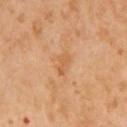Findings:
– biopsy status — total-body-photography surveillance lesion; no biopsy
– automated metrics — a normalized lesion–skin contrast near 5.5; a classifier nevus-likeness of about 0/100
– image source — 15 mm crop, total-body photography
– lesion diameter — ≈2.5 mm
– patient — male, aged 63–67